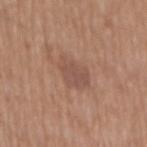– biopsy status — total-body-photography surveillance lesion; no biopsy
– patient — male, aged 68–72
– imaging modality — total-body-photography crop, ~15 mm field of view
– body site — the back
– illumination — white-light illumination
– diameter — ≈3.5 mm